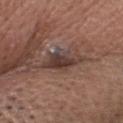Q: Is there a histopathology result?
A: catalogued during a skin exam; not biopsied
Q: Where on the body is the lesion?
A: the head or neck
Q: What is the imaging modality?
A: ~15 mm crop, total-body skin-cancer survey
Q: What are the patient's age and sex?
A: male, roughly 65 years of age